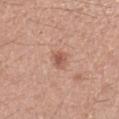Part of a total-body skin-imaging series; this lesion was reviewed on a skin check and was not flagged for biopsy. A female subject, aged approximately 40. Captured under white-light illumination. Located on the arm. The lesion's longest dimension is about 2.5 mm. An algorithmic analysis of the crop reported a nevus-likeness score of about 80/100 and a lesion-detection confidence of about 100/100. A region of skin cropped from a whole-body photographic capture, roughly 15 mm wide.